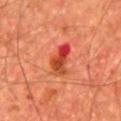workup = catalogued during a skin exam; not biopsied | body site = the chest | patient = male, aged 63–67 | acquisition = ~15 mm crop, total-body skin-cancer survey.